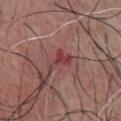This lesion was catalogued during total-body skin photography and was not selected for biopsy. A male subject, approximately 65 years of age. The tile uses white-light illumination. A 15 mm close-up tile from a total-body photography series done for melanoma screening. Located on the chest. The lesion-visualizer software estimated a lesion area of about 5 mm², a shape eccentricity near 0.85, and a shape-asymmetry score of about 0.65 (0 = symmetric). And it measured border irregularity of about 6.5 on a 0–10 scale, internal color variation of about 3 on a 0–10 scale, and a peripheral color-asymmetry measure near 1. The analysis additionally found lesion-presence confidence of about 100/100.